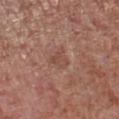| field | value |
|---|---|
| biopsy status | catalogued during a skin exam; not biopsied |
| site | the leg |
| acquisition | ~15 mm crop, total-body skin-cancer survey |
| patient | male, aged 53 to 57 |
| size | ≈2.5 mm |
| image-analysis metrics | an automated nevus-likeness rating near 0 out of 100 |
| illumination | white-light illumination |